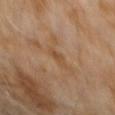| key | value |
|---|---|
| biopsy status | no biopsy performed (imaged during a skin exam) |
| tile lighting | cross-polarized |
| image | total-body-photography crop, ~15 mm field of view |
| site | the abdomen |
| patient | male, aged 58 to 62 |
| lesion diameter | ≈2.5 mm |
| automated lesion analysis | a footprint of about 2.5 mm² and an eccentricity of roughly 0.9; a mean CIELAB color near L≈46 a*≈18 b*≈32, about 6 CIELAB-L* units darker than the surrounding skin, and a lesion-to-skin contrast of about 5.5 (normalized; higher = more distinct); a border-irregularity index near 3/10, a color-variation rating of about 1/10, and a peripheral color-asymmetry measure near 0.5; a nevus-likeness score of about 0/100 and a lesion-detection confidence of about 100/100 |